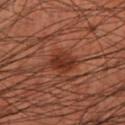Impression:
Captured during whole-body skin photography for melanoma surveillance; the lesion was not biopsied.